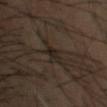Imaged during a routine full-body skin examination; the lesion was not biopsied and no histopathology is available. The tile uses cross-polarized illumination. The total-body-photography lesion software estimated about 6 CIELAB-L* units darker than the surrounding skin and a normalized border contrast of about 8.5. Cropped from a whole-body photographic skin survey; the tile spans about 15 mm. Longest diameter approximately 3 mm. A male subject aged around 45. Located on the head or neck.